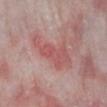The lesion was photographed on a routine skin check and not biopsied; there is no pathology result. A 15 mm close-up extracted from a 3D total-body photography capture. Captured under white-light illumination. The recorded lesion diameter is about 6.5 mm. A male subject, about 65 years old. The lesion is on the left lower leg.